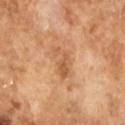Notes:
• notes · catalogued during a skin exam; not biopsied
• patient · male, in their mid- to late 60s
• tile lighting · cross-polarized illumination
• image · 15 mm crop, total-body photography
• diameter · ~3.5 mm (longest diameter)